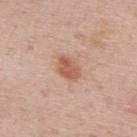Notes:
- biopsy status — no biopsy performed (imaged during a skin exam)
- anatomic site — the back
- image source — ~15 mm crop, total-body skin-cancer survey
- patient — male, aged 38–42